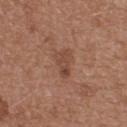Cropped from a total-body skin-imaging series; the visible field is about 15 mm. A female patient roughly 40 years of age. The tile uses white-light illumination. About 3 mm across. Located on the upper back.